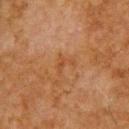Q: What is the imaging modality?
A: total-body-photography crop, ~15 mm field of view
Q: Patient demographics?
A: male, in their mid- to late 60s
Q: Where on the body is the lesion?
A: the upper back